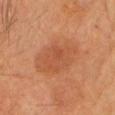  biopsy_status: not biopsied; imaged during a skin examination
  lesion_size:
    long_diameter_mm_approx: 6.5
  automated_metrics:
    eccentricity: 0.7
    shape_asymmetry: 0.15
    border_irregularity_0_10: 1.5
    color_variation_0_10: 3.0
    peripheral_color_asymmetry: 1.0
  site: head or neck
  patient:
    sex: female
    age_approx: 55
  image:
    source: total-body photography crop
    field_of_view_mm: 15
  lighting: cross-polarized